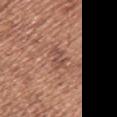Part of a total-body skin-imaging series; this lesion was reviewed on a skin check and was not flagged for biopsy.
Approximately 3 mm at its widest.
A 15 mm close-up extracted from a 3D total-body photography capture.
From the left upper arm.
A female subject approximately 50 years of age.
Captured under white-light illumination.